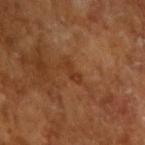Notes:
– patient: male, aged 63 to 67
– imaging modality: total-body-photography crop, ~15 mm field of view